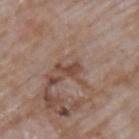Part of a total-body skin-imaging series; this lesion was reviewed on a skin check and was not flagged for biopsy. The lesion's longest dimension is about 2.5 mm. Located on the upper back. The lesion-visualizer software estimated an area of roughly 3 mm², an eccentricity of roughly 0.85, and two-axis asymmetry of about 0.65. The analysis additionally found a lesion color around L≈44 a*≈20 b*≈26 in CIELAB and a lesion–skin lightness drop of about 10. The analysis additionally found border irregularity of about 6.5 on a 0–10 scale and peripheral color asymmetry of about 0. A male subject roughly 65 years of age. Captured under white-light illumination. A roughly 15 mm field-of-view crop from a total-body skin photograph.